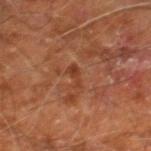Q: Was this lesion biopsied?
A: total-body-photography surveillance lesion; no biopsy
Q: What are the patient's age and sex?
A: male, roughly 60 years of age
Q: How was this image acquired?
A: ~15 mm crop, total-body skin-cancer survey
Q: What is the anatomic site?
A: the right leg
Q: Automated lesion metrics?
A: border irregularity of about 4.5 on a 0–10 scale and radial color variation of about 0
Q: Lesion size?
A: about 2.5 mm